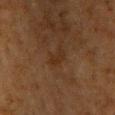Assessment:
No biopsy was performed on this lesion — it was imaged during a full skin examination and was not determined to be concerning.
Clinical summary:
A male subject approximately 60 years of age. Automated tile analysis of the lesion measured an area of roughly 3 mm² and a symmetry-axis asymmetry near 0.3. Located on the chest. The recorded lesion diameter is about 2.5 mm. Cropped from a whole-body photographic skin survey; the tile spans about 15 mm.